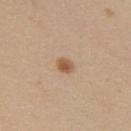This lesion was catalogued during total-body skin photography and was not selected for biopsy.
A roughly 15 mm field-of-view crop from a total-body skin photograph.
The patient is a female aged around 20.
The lesion is on the upper back.